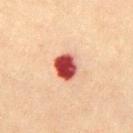This lesion was catalogued during total-body skin photography and was not selected for biopsy. On the front of the torso. The lesion's longest dimension is about 3 mm. A female subject aged 48 to 52. A close-up tile cropped from a whole-body skin photograph, about 15 mm across. The lesion-visualizer software estimated a normalized lesion–skin contrast near 16.